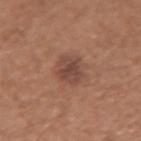Q: Was this lesion biopsied?
A: no biopsy performed (imaged during a skin exam)
Q: What did automated image analysis measure?
A: an area of roughly 8.5 mm², a shape eccentricity near 0.55, and a shape-asymmetry score of about 0.25 (0 = symmetric); a mean CIELAB color near L≈45 a*≈20 b*≈26, about 9 CIELAB-L* units darker than the surrounding skin, and a normalized border contrast of about 7.5; a border-irregularity rating of about 2.5/10 and a within-lesion color-variation index near 3.5/10; a classifier nevus-likeness of about 35/100 and a detector confidence of about 100 out of 100 that the crop contains a lesion
Q: What is the lesion's diameter?
A: ~4 mm (longest diameter)
Q: Where on the body is the lesion?
A: the left forearm
Q: How was the tile lit?
A: white-light
Q: Who is the patient?
A: female, aged around 40
Q: How was this image acquired?
A: 15 mm crop, total-body photography